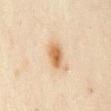Clinical impression: The lesion was tiled from a total-body skin photograph and was not biopsied. Context: A female patient aged approximately 35. A region of skin cropped from a whole-body photographic capture, roughly 15 mm wide. The lesion-visualizer software estimated a footprint of about 6 mm², an eccentricity of roughly 0.75, and two-axis asymmetry of about 0.2. And it measured an average lesion color of about L≈64 a*≈19 b*≈38 (CIELAB), about 12 CIELAB-L* units darker than the surrounding skin, and a normalized lesion–skin contrast near 9. The software also gave a detector confidence of about 100 out of 100 that the crop contains a lesion. Measured at roughly 3.5 mm in maximum diameter. The tile uses cross-polarized illumination. The lesion is on the mid back.